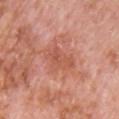This lesion was catalogued during total-body skin photography and was not selected for biopsy. From the chest. Cropped from a total-body skin-imaging series; the visible field is about 15 mm. The recorded lesion diameter is about 4.5 mm. The tile uses white-light illumination. A male patient, aged 78–82. The lesion-visualizer software estimated an eccentricity of roughly 0.8 and a symmetry-axis asymmetry near 0.45. And it measured roughly 7 lightness units darker than nearby skin and a normalized border contrast of about 5.5.